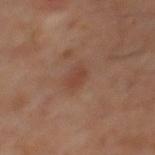Notes:
– biopsy status — no biopsy performed (imaged during a skin exam)
– imaging modality — ~15 mm crop, total-body skin-cancer survey
– subject — male, aged around 60
– diameter — ~2.5 mm (longest diameter)
– TBP lesion metrics — a lesion area of about 2.5 mm², an outline eccentricity of about 0.9 (0 = round, 1 = elongated), and a shape-asymmetry score of about 0.4 (0 = symmetric); a lesion–skin lightness drop of about 6 and a normalized border contrast of about 5.5; a border-irregularity rating of about 3.5/10, internal color variation of about 1.5 on a 0–10 scale, and a peripheral color-asymmetry measure near 0.5
– illumination — cross-polarized illumination
– anatomic site — the mid back